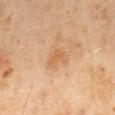Assessment:
Imaged during a routine full-body skin examination; the lesion was not biopsied and no histopathology is available.
Clinical summary:
Captured under cross-polarized illumination. The recorded lesion diameter is about 3 mm. The patient is a male aged around 45. The lesion is on the mid back. A region of skin cropped from a whole-body photographic capture, roughly 15 mm wide.